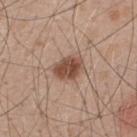Q: Is there a histopathology result?
A: no biopsy performed (imaged during a skin exam)
Q: What are the patient's age and sex?
A: male, in their 50s
Q: What is the imaging modality?
A: 15 mm crop, total-body photography
Q: Where on the body is the lesion?
A: the upper back